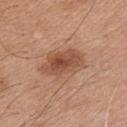This lesion was catalogued during total-body skin photography and was not selected for biopsy. Cropped from a whole-body photographic skin survey; the tile spans about 15 mm. The recorded lesion diameter is about 5.5 mm. The subject is a male aged 43–47. The lesion is located on the chest. Automated image analysis of the tile measured a shape eccentricity near 0.85 and two-axis asymmetry of about 0.2. The tile uses white-light illumination.